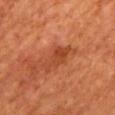| key | value |
|---|---|
| workup | no biopsy performed (imaged during a skin exam) |
| patient | male, in their mid-60s |
| size | about 4 mm |
| body site | the mid back |
| tile lighting | cross-polarized illumination |
| automated lesion analysis | a lesion area of about 6 mm², a shape eccentricity near 0.8, and a symmetry-axis asymmetry near 0.35; a mean CIELAB color near L≈40 a*≈28 b*≈35, about 7 CIELAB-L* units darker than the surrounding skin, and a normalized border contrast of about 6; an automated nevus-likeness rating near 0 out of 100 and a detector confidence of about 100 out of 100 that the crop contains a lesion |
| image | 15 mm crop, total-body photography |